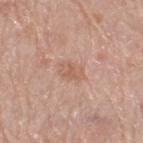biopsy status — catalogued during a skin exam; not biopsied
tile lighting — white-light
size — about 3 mm
imaging modality — ~15 mm crop, total-body skin-cancer survey
subject — male, aged 78 to 82
automated metrics — a lesion color around L≈59 a*≈21 b*≈29 in CIELAB; internal color variation of about 3 on a 0–10 scale and radial color variation of about 1; an automated nevus-likeness rating near 0 out of 100
anatomic site — the right thigh